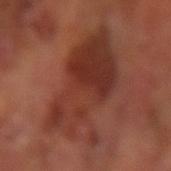This lesion was catalogued during total-body skin photography and was not selected for biopsy.
A region of skin cropped from a whole-body photographic capture, roughly 15 mm wide.
From the left arm.
Longest diameter approximately 10 mm.
The tile uses cross-polarized illumination.
A male subject aged approximately 65.